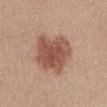Impression: Part of a total-body skin-imaging series; this lesion was reviewed on a skin check and was not flagged for biopsy. Clinical summary: Approximately 6 mm at its widest. A female subject in their mid-40s. Imaged with white-light lighting. An algorithmic analysis of the crop reported a border-irregularity rating of about 2.5/10. A 15 mm close-up tile from a total-body photography series done for melanoma screening. The lesion is on the abdomen.